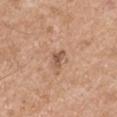workup — imaged on a skin check; not biopsied
automated lesion analysis — a footprint of about 3 mm², a shape eccentricity near 0.8, and a shape-asymmetry score of about 0.45 (0 = symmetric); a lesion-detection confidence of about 100/100
illumination — white-light
body site — the right upper arm
diameter — ~2.5 mm (longest diameter)
image — ~15 mm crop, total-body skin-cancer survey
patient — male, aged around 55